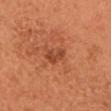Context: About 2.5 mm across. Automated image analysis of the tile measured a lesion area of about 4.5 mm², an eccentricity of roughly 0.65, and a symmetry-axis asymmetry near 0.35. It also reported border irregularity of about 3.5 on a 0–10 scale. The subject is a female in their mid-50s. A 15 mm crop from a total-body photograph taken for skin-cancer surveillance. On the head or neck. The tile uses cross-polarized illumination.